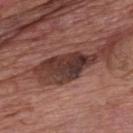Imaged during a routine full-body skin examination; the lesion was not biopsied and no histopathology is available. The lesion-visualizer software estimated a lesion area of about 14 mm², an eccentricity of roughly 0.85, and two-axis asymmetry of about 0.35. The analysis additionally found a mean CIELAB color near L≈34 a*≈20 b*≈21, roughly 15 lightness units darker than nearby skin, and a normalized border contrast of about 12. The software also gave a lesion-detection confidence of about 85/100. A 15 mm close-up tile from a total-body photography series done for melanoma screening. Approximately 7 mm at its widest. A male patient, approximately 75 years of age. The lesion is located on the chest.